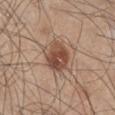Q: Is there a histopathology result?
A: no biopsy performed (imaged during a skin exam)
Q: What are the patient's age and sex?
A: male, aged around 45
Q: Where on the body is the lesion?
A: the leg
Q: How was the tile lit?
A: white-light illumination
Q: What kind of image is this?
A: total-body-photography crop, ~15 mm field of view
Q: What is the lesion's diameter?
A: ~3.5 mm (longest diameter)
Q: What did automated image analysis measure?
A: a footprint of about 10 mm² and a shape eccentricity near 0.35; about 12 CIELAB-L* units darker than the surrounding skin and a lesion-to-skin contrast of about 9 (normalized; higher = more distinct)